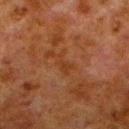Recorded during total-body skin imaging; not selected for excision or biopsy. The subject is a male aged 78–82. This image is a 15 mm lesion crop taken from a total-body photograph. The lesion is on the left lower leg. Automated image analysis of the tile measured a lesion area of about 3.5 mm², an eccentricity of roughly 0.9, and a shape-asymmetry score of about 0.5 (0 = symmetric). And it measured a lesion–skin lightness drop of about 4 and a normalized lesion–skin contrast near 5. It also reported a border-irregularity rating of about 6/10, a within-lesion color-variation index near 0.5/10, and a peripheral color-asymmetry measure near 0.